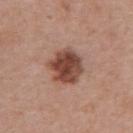notes: total-body-photography surveillance lesion; no biopsy
subject: male, approximately 70 years of age
size: ~5 mm (longest diameter)
imaging modality: total-body-photography crop, ~15 mm field of view
automated metrics: a footprint of about 13 mm², an outline eccentricity of about 0.65 (0 = round, 1 = elongated), and a symmetry-axis asymmetry near 0.15; an average lesion color of about L≈45 a*≈22 b*≈27 (CIELAB), about 15 CIELAB-L* units darker than the surrounding skin, and a lesion-to-skin contrast of about 11 (normalized; higher = more distinct); a peripheral color-asymmetry measure near 1.5; a classifier nevus-likeness of about 70/100
lighting: white-light
site: the abdomen